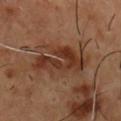This lesion was catalogued during total-body skin photography and was not selected for biopsy.